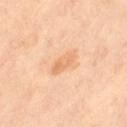Q: Is there a histopathology result?
A: no biopsy performed (imaged during a skin exam)
Q: Lesion location?
A: the right thigh
Q: What are the patient's age and sex?
A: female, aged 48–52
Q: What is the imaging modality?
A: ~15 mm tile from a whole-body skin photo
Q: Automated lesion metrics?
A: an average lesion color of about L≈73 a*≈23 b*≈40 (CIELAB) and roughly 8 lightness units darker than nearby skin; border irregularity of about 3.5 on a 0–10 scale and peripheral color asymmetry of about 1.5
Q: How large is the lesion?
A: ~3 mm (longest diameter)